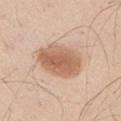{
  "biopsy_status": "not biopsied; imaged during a skin examination",
  "patient": {
    "sex": "male",
    "age_approx": 35
  },
  "image": {
    "source": "total-body photography crop",
    "field_of_view_mm": 15
  },
  "lesion_size": {
    "long_diameter_mm_approx": 6.0
  },
  "site": "right upper arm",
  "lighting": "white-light"
}